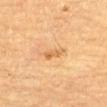Imaged during a routine full-body skin examination; the lesion was not biopsied and no histopathology is available. The lesion is located on the front of the torso. The total-body-photography lesion software estimated a lesion color around L≈57 a*≈20 b*≈39 in CIELAB, roughly 8 lightness units darker than nearby skin, and a normalized lesion–skin contrast near 6. The software also gave border irregularity of about 3.5 on a 0–10 scale and internal color variation of about 2 on a 0–10 scale. The analysis additionally found a classifier nevus-likeness of about 5/100 and a detector confidence of about 100 out of 100 that the crop contains a lesion. Cropped from a whole-body photographic skin survey; the tile spans about 15 mm. About 2.5 mm across. A male subject aged 83 or older.